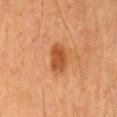biopsy_status: not biopsied; imaged during a skin examination
lesion_size:
  long_diameter_mm_approx: 4.0
patient:
  sex: male
  age_approx: 65
image:
  source: total-body photography crop
  field_of_view_mm: 15
site: mid back